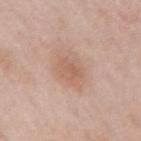Impression: This lesion was catalogued during total-body skin photography and was not selected for biopsy. Clinical summary: The lesion is on the right upper arm. This is a white-light tile. The lesion-visualizer software estimated a border-irregularity rating of about 2.5/10 and a peripheral color-asymmetry measure near 0.5. A 15 mm close-up extracted from a 3D total-body photography capture. The subject is a male aged 63 to 67. About 3 mm across.